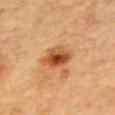This lesion was catalogued during total-body skin photography and was not selected for biopsy.
A male patient in their mid-80s or older.
On the chest.
A 15 mm close-up tile from a total-body photography series done for melanoma screening.
The recorded lesion diameter is about 4 mm.